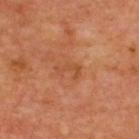Clinical summary: The patient is aged around 65. The total-body-photography lesion software estimated a shape eccentricity near 0.85 and two-axis asymmetry of about 0.55. The recorded lesion diameter is about 3 mm. The tile uses cross-polarized illumination. Cropped from a total-body skin-imaging series; the visible field is about 15 mm. The lesion is on the upper back.